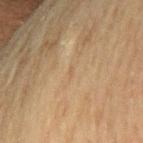Captured during whole-body skin photography for melanoma surveillance; the lesion was not biopsied. The recorded lesion diameter is about 1 mm. Captured under cross-polarized illumination. The lesion-visualizer software estimated a lesion color around L≈50 a*≈13 b*≈32 in CIELAB, a lesion–skin lightness drop of about 4, and a normalized border contrast of about 3.5. The software also gave an automated nevus-likeness rating near 0 out of 100. Located on the right upper arm. A region of skin cropped from a whole-body photographic capture, roughly 15 mm wide. A female subject aged approximately 80.